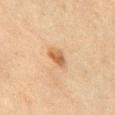biopsy status=total-body-photography surveillance lesion; no biopsy | subject=male, aged approximately 70 | imaging modality=~15 mm crop, total-body skin-cancer survey | site=the chest | automated metrics=a lesion area of about 4 mm², an eccentricity of roughly 0.75, and a symmetry-axis asymmetry near 0.3; a mean CIELAB color near L≈50 a*≈18 b*≈34 and roughly 10 lightness units darker than nearby skin; a within-lesion color-variation index near 3/10 and a peripheral color-asymmetry measure near 1; a classifier nevus-likeness of about 85/100 and a detector confidence of about 100 out of 100 that the crop contains a lesion | tile lighting=cross-polarized.